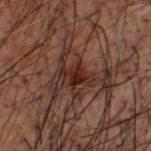Q: Was this lesion biopsied?
A: imaged on a skin check; not biopsied
Q: What are the patient's age and sex?
A: male, about 50 years old
Q: Automated lesion metrics?
A: an area of roughly 5 mm² and a shape-asymmetry score of about 0.3 (0 = symmetric)
Q: How was this image acquired?
A: ~15 mm tile from a whole-body skin photo
Q: What is the anatomic site?
A: the head or neck
Q: Lesion size?
A: about 3 mm